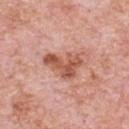<case>
  <biopsy_status>not biopsied; imaged during a skin examination</biopsy_status>
  <patient>
    <sex>male</sex>
    <age_approx>80</age_approx>
  </patient>
  <lesion_size>
    <long_diameter_mm_approx>5.0</long_diameter_mm_approx>
  </lesion_size>
  <site>chest</site>
  <image>
    <source>total-body photography crop</source>
    <field_of_view_mm>15</field_of_view_mm>
  </image>
  <automated_metrics>
    <area_mm2_approx>10.0</area_mm2_approx>
    <shape_asymmetry>0.5</shape_asymmetry>
    <cielab_L>55</cielab_L>
    <cielab_a>26</cielab_a>
    <cielab_b>31</cielab_b>
    <vs_skin_contrast_norm>8.5</vs_skin_contrast_norm>
    <color_variation_0_10>4.5</color_variation_0_10>
    <lesion_detection_confidence_0_100>100</lesion_detection_confidence_0_100>
  </automated_metrics>
  <lighting>white-light</lighting>
</case>